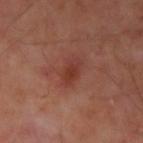Acquisition and patient details: A region of skin cropped from a whole-body photographic capture, roughly 15 mm wide. Located on the left forearm. The subject is a male in their 50s. Measured at roughly 3.5 mm in maximum diameter.